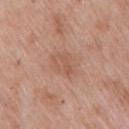• location · the right upper arm
• image source · ~15 mm tile from a whole-body skin photo
• automated metrics · an area of roughly 8.5 mm² and a shape eccentricity near 0.65; an average lesion color of about L≈56 a*≈20 b*≈29 (CIELAB), a lesion–skin lightness drop of about 6, and a lesion-to-skin contrast of about 4.5 (normalized; higher = more distinct); a border-irregularity rating of about 2.5/10, a color-variation rating of about 2.5/10, and radial color variation of about 1; a nevus-likeness score of about 0/100 and a lesion-detection confidence of about 100/100
• subject · female, roughly 70 years of age